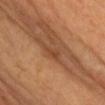No biopsy was performed on this lesion — it was imaged during a full skin examination and was not determined to be concerning. The subject is a female in their 60s. The lesion is on the head or neck. This is a cross-polarized tile. A 15 mm close-up tile from a total-body photography series done for melanoma screening. Approximately 3 mm at its widest.